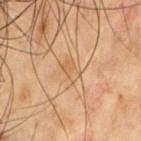{
  "biopsy_status": "not biopsied; imaged during a skin examination",
  "lesion_size": {
    "long_diameter_mm_approx": 2.5
  },
  "patient": {
    "sex": "male",
    "age_approx": 55
  },
  "image": {
    "source": "total-body photography crop",
    "field_of_view_mm": 15
  },
  "automated_metrics": {
    "area_mm2_approx": 3.5,
    "eccentricity": 0.8,
    "shape_asymmetry": 0.3,
    "border_irregularity_0_10": 2.5,
    "color_variation_0_10": 3.0,
    "peripheral_color_asymmetry": 1.0,
    "nevus_likeness_0_100": 0,
    "lesion_detection_confidence_0_100": 100
  },
  "site": "chest"
}